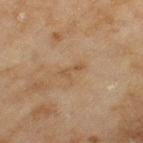| field | value |
|---|---|
| biopsy status | no biopsy performed (imaged during a skin exam) |
| lighting | cross-polarized |
| image | ~15 mm crop, total-body skin-cancer survey |
| size | about 2.5 mm |
| location | the left thigh |
| subject | female, about 60 years old |